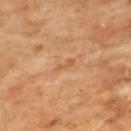follow-up: total-body-photography surveillance lesion; no biopsy
anatomic site: the upper back
lighting: cross-polarized
size: about 3 mm
image source: total-body-photography crop, ~15 mm field of view